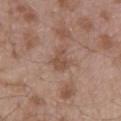Acquisition and patient details: The recorded lesion diameter is about 2.5 mm. A region of skin cropped from a whole-body photographic capture, roughly 15 mm wide. A male patient in their mid- to late 50s. On the lower back. Imaged with white-light lighting.